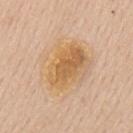biopsy status: catalogued during a skin exam; not biopsied
illumination: white-light
image: 15 mm crop, total-body photography
automated metrics: a lesion area of about 34 mm² and a shape eccentricity near 0.55; a mean CIELAB color near L≈66 a*≈17 b*≈38, a lesion–skin lightness drop of about 7, and a lesion-to-skin contrast of about 7 (normalized; higher = more distinct); a border-irregularity index near 2/10 and a color-variation rating of about 8/10
subject: male, aged around 60
site: the upper back